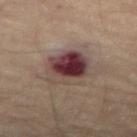{"biopsy_status": "not biopsied; imaged during a skin examination", "site": "right thigh", "lighting": "cross-polarized", "image": {"source": "total-body photography crop", "field_of_view_mm": 15}, "automated_metrics": {"area_mm2_approx": 16.0, "eccentricity": 0.4, "shape_asymmetry": 0.15, "nevus_likeness_0_100": 0, "lesion_detection_confidence_0_100": 100}, "lesion_size": {"long_diameter_mm_approx": 5.0}, "patient": {"sex": "male", "age_approx": 65}}